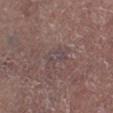Part of a total-body skin-imaging series; this lesion was reviewed on a skin check and was not flagged for biopsy. A male subject, in their mid-50s. A roughly 15 mm field-of-view crop from a total-body skin photograph. The recorded lesion diameter is about 2.5 mm. Automated image analysis of the tile measured a lesion–skin lightness drop of about 5 and a normalized border contrast of about 5.5. The software also gave a nevus-likeness score of about 0/100 and a detector confidence of about 65 out of 100 that the crop contains a lesion. The tile uses white-light illumination. On the right lower leg.